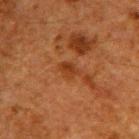Impression:
Captured during whole-body skin photography for melanoma surveillance; the lesion was not biopsied.
Context:
This image is a 15 mm lesion crop taken from a total-body photograph. The patient is a male aged 58–62. On the right upper arm. The lesion's longest dimension is about 2.5 mm.